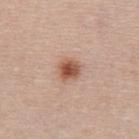Clinical impression:
The lesion was photographed on a routine skin check and not biopsied; there is no pathology result.
Context:
From the upper back. The total-body-photography lesion software estimated an outline eccentricity of about 0.35 (0 = round, 1 = elongated) and two-axis asymmetry of about 0.2. And it measured an average lesion color of about L≈54 a*≈23 b*≈30 (CIELAB). It also reported border irregularity of about 1.5 on a 0–10 scale, a color-variation rating of about 4.5/10, and peripheral color asymmetry of about 1. About 2.5 mm across. A female subject, approximately 55 years of age. This image is a 15 mm lesion crop taken from a total-body photograph.